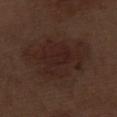Impression:
Part of a total-body skin-imaging series; this lesion was reviewed on a skin check and was not flagged for biopsy.
Acquisition and patient details:
The lesion is on the left thigh. The subject is a male approximately 70 years of age. The recorded lesion diameter is about 8 mm. This is a white-light tile. A 15 mm crop from a total-body photograph taken for skin-cancer surveillance.